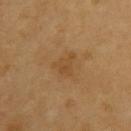<tbp_lesion>
<biopsy_status>not biopsied; imaged during a skin examination</biopsy_status>
<automated_metrics>
  <area_mm2_approx>4.5</area_mm2_approx>
  <eccentricity>0.7</eccentricity>
  <cielab_L>46</cielab_L>
  <cielab_a>18</cielab_a>
  <cielab_b>37</cielab_b>
  <vs_skin_contrast_norm>5.0</vs_skin_contrast_norm>
  <nevus_likeness_0_100>0</nevus_likeness_0_100>
  <lesion_detection_confidence_0_100>100</lesion_detection_confidence_0_100>
</automated_metrics>
<site>back</site>
<image>
  <source>total-body photography crop</source>
  <field_of_view_mm>15</field_of_view_mm>
</image>
<patient>
  <sex>male</sex>
  <age_approx>60</age_approx>
</patient>
<lighting>cross-polarized</lighting>
</tbp_lesion>